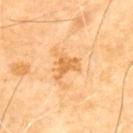<record>
  <biopsy_status>not biopsied; imaged during a skin examination</biopsy_status>
  <patient>
    <sex>male</sex>
    <age_approx>70</age_approx>
  </patient>
  <lighting>cross-polarized</lighting>
  <image>
    <source>total-body photography crop</source>
    <field_of_view_mm>15</field_of_view_mm>
  </image>
  <automated_metrics>
    <border_irregularity_0_10>5.5</border_irregularity_0_10>
    <color_variation_0_10>1.5</color_variation_0_10>
    <peripheral_color_asymmetry>0.5</peripheral_color_asymmetry>
  </automated_metrics>
  <lesion_size>
    <long_diameter_mm_approx>3.5</long_diameter_mm_approx>
  </lesion_size>
  <site>upper back</site>
</record>